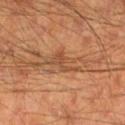Clinical impression:
The lesion was photographed on a routine skin check and not biopsied; there is no pathology result.
Context:
The lesion is located on the left lower leg. Cropped from a total-body skin-imaging series; the visible field is about 15 mm. A male patient aged approximately 65.